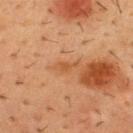Assessment: Captured during whole-body skin photography for melanoma surveillance; the lesion was not biopsied. Acquisition and patient details: This is a cross-polarized tile. A close-up tile cropped from a whole-body skin photograph, about 15 mm across. A male patient in their mid-50s. The lesion is located on the chest.